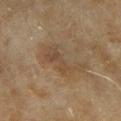Located on the right upper arm. Cropped from a total-body skin-imaging series; the visible field is about 15 mm. The tile uses cross-polarized illumination. A female subject aged around 60.